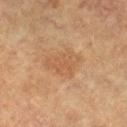Clinical impression:
Imaged during a routine full-body skin examination; the lesion was not biopsied and no histopathology is available.
Context:
A 15 mm crop from a total-body photograph taken for skin-cancer surveillance. The tile uses cross-polarized illumination. The lesion's longest dimension is about 4.5 mm. On the right lower leg. The patient is a female about 60 years old.